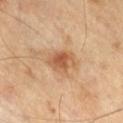This lesion was catalogued during total-body skin photography and was not selected for biopsy. Approximately 3.5 mm at its widest. A lesion tile, about 15 mm wide, cut from a 3D total-body photograph. Located on the leg. This is a cross-polarized tile. A male subject aged around 70.